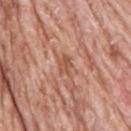Clinical impression: Part of a total-body skin-imaging series; this lesion was reviewed on a skin check and was not flagged for biopsy. Background: Cropped from a total-body skin-imaging series; the visible field is about 15 mm. Located on the head or neck. The lesion's longest dimension is about 2.5 mm. Imaged with white-light lighting. Automated image analysis of the tile measured a mean CIELAB color near L≈54 a*≈24 b*≈31 and about 9 CIELAB-L* units darker than the surrounding skin. The software also gave radial color variation of about 1.5. The software also gave a nevus-likeness score of about 0/100. The subject is a male about 75 years old.